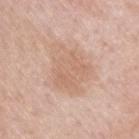site: back
image:
  source: total-body photography crop
  field_of_view_mm: 15
patient:
  sex: male
  age_approx: 60